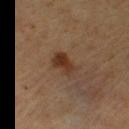Recorded during total-body skin imaging; not selected for excision or biopsy.
A roughly 15 mm field-of-view crop from a total-body skin photograph.
Measured at roughly 3 mm in maximum diameter.
A female subject aged around 40.
Captured under cross-polarized illumination.
From the right thigh.
The total-body-photography lesion software estimated an area of roughly 6 mm², a shape eccentricity near 0.65, and a shape-asymmetry score of about 0.3 (0 = symmetric). And it measured border irregularity of about 3 on a 0–10 scale, internal color variation of about 4 on a 0–10 scale, and peripheral color asymmetry of about 1.5.